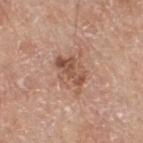The lesion is on the upper back. This image is a 15 mm lesion crop taken from a total-body photograph. This is a white-light tile. The lesion's longest dimension is about 5 mm. An algorithmic analysis of the crop reported an area of roughly 11 mm² and an outline eccentricity of about 0.6 (0 = round, 1 = elongated). It also reported a lesion color around L≈54 a*≈21 b*≈29 in CIELAB and about 10 CIELAB-L* units darker than the surrounding skin. And it measured a border-irregularity index near 5/10, a within-lesion color-variation index near 6/10, and a peripheral color-asymmetry measure near 2. The subject is a male aged 78 to 82.